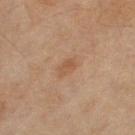patient: female, aged approximately 60 | diameter: ≈2.5 mm | illumination: cross-polarized illumination | anatomic site: the right thigh | image-analysis metrics: a footprint of about 3.5 mm², a shape eccentricity near 0.85, and a shape-asymmetry score of about 0.25 (0 = symmetric); a lesion color around L≈48 a*≈18 b*≈30 in CIELAB and a normalized border contrast of about 5.5; a classifier nevus-likeness of about 30/100 and a detector confidence of about 100 out of 100 that the crop contains a lesion | imaging modality: ~15 mm tile from a whole-body skin photo.